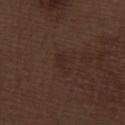Acquisition and patient details: On the right thigh. A close-up tile cropped from a whole-body skin photograph, about 15 mm across. Automated image analysis of the tile measured a classifier nevus-likeness of about 20/100. A male subject roughly 70 years of age.